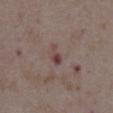workup = total-body-photography surveillance lesion; no biopsy | patient = male, aged 73 to 77 | anatomic site = the abdomen | lighting = white-light | acquisition = ~15 mm crop, total-body skin-cancer survey | size = ~2.5 mm (longest diameter).